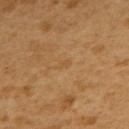biopsy_status: not biopsied; imaged during a skin examination
site: back
patient:
  sex: female
  age_approx: 55
image:
  source: total-body photography crop
  field_of_view_mm: 15